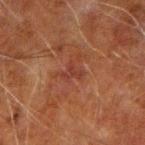patient = male, approximately 60 years of age
anatomic site = the arm
image = total-body-photography crop, ~15 mm field of view